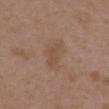Assessment:
This lesion was catalogued during total-body skin photography and was not selected for biopsy.
Image and clinical context:
Measured at roughly 4.5 mm in maximum diameter. The patient is a female roughly 35 years of age. The lesion is on the upper back. This is a white-light tile. A close-up tile cropped from a whole-body skin photograph, about 15 mm across.